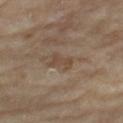{
  "biopsy_status": "not biopsied; imaged during a skin examination",
  "lesion_size": {
    "long_diameter_mm_approx": 3.0
  },
  "site": "right thigh",
  "automated_metrics": {
    "cielab_L": 45,
    "cielab_a": 14,
    "cielab_b": 27,
    "vs_skin_darker_L": 7.0,
    "vs_skin_contrast_norm": 6.0,
    "border_irregularity_0_10": 5.0,
    "color_variation_0_10": 0.0,
    "peripheral_color_asymmetry": 0.0,
    "nevus_likeness_0_100": 0,
    "lesion_detection_confidence_0_100": 95
  },
  "patient": {
    "sex": "female",
    "age_approx": 75
  },
  "image": {
    "source": "total-body photography crop",
    "field_of_view_mm": 15
  },
  "lighting": "cross-polarized"
}